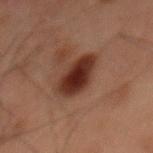Clinical summary: A male patient aged around 60. A 15 mm close-up extracted from a 3D total-body photography capture. Measured at roughly 5 mm in maximum diameter. Automated image analysis of the tile measured a border-irregularity index near 2/10, a color-variation rating of about 3.5/10, and peripheral color asymmetry of about 1. The tile uses cross-polarized illumination. The lesion is on the mid back.